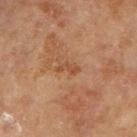{
  "biopsy_status": "not biopsied; imaged during a skin examination",
  "patient": {
    "sex": "female",
    "age_approx": 65
  },
  "lighting": "cross-polarized",
  "site": "left arm",
  "lesion_size": {
    "long_diameter_mm_approx": 3.0
  },
  "image": {
    "source": "total-body photography crop",
    "field_of_view_mm": 15
  }
}